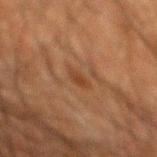<case>
  <biopsy_status>not biopsied; imaged during a skin examination</biopsy_status>
  <image>
    <source>total-body photography crop</source>
    <field_of_view_mm>15</field_of_view_mm>
  </image>
  <lighting>cross-polarized</lighting>
  <site>mid back</site>
  <lesion_size>
    <long_diameter_mm_approx>3.0</long_diameter_mm_approx>
  </lesion_size>
  <patient>
    <sex>male</sex>
    <age_approx>60</age_approx>
  </patient>
</case>